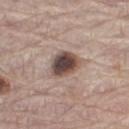Impression:
The lesion was photographed on a routine skin check and not biopsied; there is no pathology result.
Acquisition and patient details:
A close-up tile cropped from a whole-body skin photograph, about 15 mm across. Located on the right thigh. About 3.5 mm across. A male subject, in their mid- to late 60s. The tile uses white-light illumination. The total-body-photography lesion software estimated an area of roughly 9 mm², a shape eccentricity near 0.6, and a shape-asymmetry score of about 0.2 (0 = symmetric). It also reported border irregularity of about 2 on a 0–10 scale and internal color variation of about 7.5 on a 0–10 scale. The software also gave an automated nevus-likeness rating near 95 out of 100 and lesion-presence confidence of about 100/100.